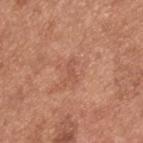- workup — imaged on a skin check; not biopsied
- illumination — white-light illumination
- subject — male, aged approximately 55
- location — the upper back
- imaging modality — ~15 mm crop, total-body skin-cancer survey
- lesion diameter — ~2.5 mm (longest diameter)